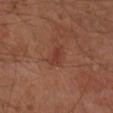biopsy status — total-body-photography surveillance lesion; no biopsy | image source — ~15 mm crop, total-body skin-cancer survey | anatomic site — the right forearm | size — ~3 mm (longest diameter) | illumination — cross-polarized illumination | automated lesion analysis — a lesion area of about 3.5 mm², a shape eccentricity near 0.9, and a symmetry-axis asymmetry near 0.35; a nevus-likeness score of about 20/100 and lesion-presence confidence of about 100/100 | subject — approximately 55 years of age.